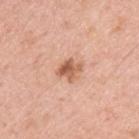Imaged during a routine full-body skin examination; the lesion was not biopsied and no histopathology is available. A male subject, aged approximately 65. The recorded lesion diameter is about 3 mm. The total-body-photography lesion software estimated border irregularity of about 2.5 on a 0–10 scale, internal color variation of about 6 on a 0–10 scale, and peripheral color asymmetry of about 2.5. It also reported an automated nevus-likeness rating near 55 out of 100 and lesion-presence confidence of about 100/100. This is a white-light tile. A roughly 15 mm field-of-view crop from a total-body skin photograph. The lesion is on the upper back.